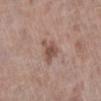Impression:
Part of a total-body skin-imaging series; this lesion was reviewed on a skin check and was not flagged for biopsy.
Image and clinical context:
From the left lower leg. The tile uses white-light illumination. Cropped from a whole-body photographic skin survey; the tile spans about 15 mm. The patient is a female approximately 70 years of age. The lesion's longest dimension is about 3 mm. Automated tile analysis of the lesion measured a lesion area of about 5.5 mm² and a symmetry-axis asymmetry near 0.45.